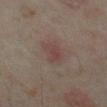Findings:
• patient · female, approximately 35 years of age
• size · about 3 mm
• anatomic site · the left lower leg
• image · total-body-photography crop, ~15 mm field of view